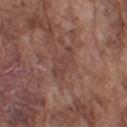Impression:
Captured during whole-body skin photography for melanoma surveillance; the lesion was not biopsied.
Clinical summary:
A male patient roughly 75 years of age. The lesion's longest dimension is about 4 mm. Cropped from a whole-body photographic skin survey; the tile spans about 15 mm. The total-body-photography lesion software estimated a shape eccentricity near 0.9 and a shape-asymmetry score of about 0.4 (0 = symmetric). It also reported about 6 CIELAB-L* units darker than the surrounding skin. Located on the left upper arm.